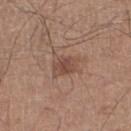Q: Is there a histopathology result?
A: no biopsy performed (imaged during a skin exam)
Q: Illumination type?
A: white-light illumination
Q: Who is the patient?
A: male, approximately 60 years of age
Q: What kind of image is this?
A: ~15 mm crop, total-body skin-cancer survey
Q: Lesion size?
A: ~3.5 mm (longest diameter)
Q: Where on the body is the lesion?
A: the right lower leg